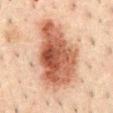Q: Was this lesion biopsied?
A: total-body-photography surveillance lesion; no biopsy
Q: What lighting was used for the tile?
A: cross-polarized
Q: What is the anatomic site?
A: the abdomen
Q: What are the patient's age and sex?
A: male, aged 48 to 52
Q: What kind of image is this?
A: 15 mm crop, total-body photography